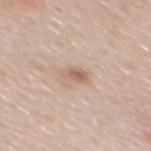Findings:
– notes · catalogued during a skin exam; not biopsied
– patient · male, roughly 55 years of age
– tile lighting · white-light
– imaging modality · 15 mm crop, total-body photography
– lesion diameter · about 2.5 mm
– location · the mid back